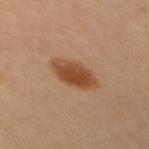The lesion was photographed on a routine skin check and not biopsied; there is no pathology result. A 15 mm close-up tile from a total-body photography series done for melanoma screening. The lesion-visualizer software estimated a lesion area of about 10 mm² and an eccentricity of roughly 0.8. The tile uses cross-polarized illumination. Longest diameter approximately 5 mm. The patient is a female in their 50s.